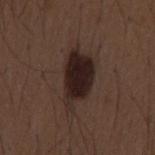Imaged during a routine full-body skin examination; the lesion was not biopsied and no histopathology is available. A male subject, aged approximately 50. The lesion is located on the mid back. A roughly 15 mm field-of-view crop from a total-body skin photograph. Captured under white-light illumination. The lesion's longest dimension is about 6.5 mm.